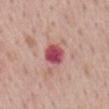This lesion was catalogued during total-body skin photography and was not selected for biopsy.
A female patient in their mid- to late 60s.
The lesion is on the mid back.
A 15 mm close-up tile from a total-body photography series done for melanoma screening.